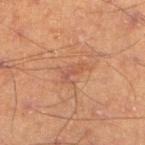{
  "biopsy_status": "not biopsied; imaged during a skin examination",
  "lesion_size": {
    "long_diameter_mm_approx": 2.5
  },
  "image": {
    "source": "total-body photography crop",
    "field_of_view_mm": 15
  },
  "automated_metrics": {
    "area_mm2_approx": 2.0,
    "eccentricity": 0.95,
    "border_irregularity_0_10": 5.5,
    "color_variation_0_10": 0.0,
    "peripheral_color_asymmetry": 0.0
  },
  "patient": {
    "sex": "male",
    "age_approx": 55
  },
  "site": "right lower leg",
  "lighting": "cross-polarized"
}